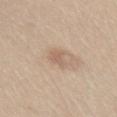workup = catalogued during a skin exam; not biopsied
site = the abdomen
imaging modality = 15 mm crop, total-body photography
lesion size = about 3 mm
patient = female, roughly 45 years of age